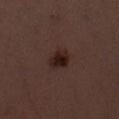Q: What did automated image analysis measure?
A: a footprint of about 5.5 mm², an outline eccentricity of about 0.6 (0 = round, 1 = elongated), and a shape-asymmetry score of about 0.2 (0 = symmetric); a lesion–skin lightness drop of about 9 and a normalized border contrast of about 11; a border-irregularity index near 1.5/10 and peripheral color asymmetry of about 1
Q: What kind of image is this?
A: 15 mm crop, total-body photography
Q: Lesion size?
A: ~2.5 mm (longest diameter)
Q: How was the tile lit?
A: white-light
Q: Patient demographics?
A: female, about 50 years old
Q: Where on the body is the lesion?
A: the right thigh